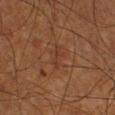– follow-up — total-body-photography surveillance lesion; no biopsy
– automated lesion analysis — an average lesion color of about L≈35 a*≈21 b*≈29 (CIELAB), about 5 CIELAB-L* units darker than the surrounding skin, and a normalized lesion–skin contrast near 5; a classifier nevus-likeness of about 0/100 and lesion-presence confidence of about 100/100
– anatomic site — the leg
– image source — ~15 mm tile from a whole-body skin photo
– tile lighting — cross-polarized illumination
– patient — male, about 70 years old